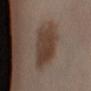Assessment: Imaged during a routine full-body skin examination; the lesion was not biopsied and no histopathology is available. Image and clinical context: A female patient approximately 50 years of age. A lesion tile, about 15 mm wide, cut from a 3D total-body photograph. Located on the left lower leg. About 6 mm across. The lesion-visualizer software estimated a border-irregularity rating of about 2/10, internal color variation of about 3.5 on a 0–10 scale, and peripheral color asymmetry of about 1. The software also gave a nevus-likeness score of about 95/100 and a detector confidence of about 100 out of 100 that the crop contains a lesion.